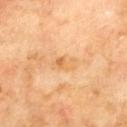Q: What are the patient's age and sex?
A: male, aged approximately 70
Q: What did automated image analysis measure?
A: an automated nevus-likeness rating near 0 out of 100
Q: What kind of image is this?
A: 15 mm crop, total-body photography
Q: Lesion location?
A: the mid back